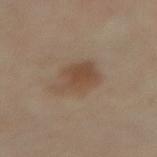No biopsy was performed on this lesion — it was imaged during a full skin examination and was not determined to be concerning. A 15 mm close-up tile from a total-body photography series done for melanoma screening. Located on the right thigh. A female subject aged around 50. Measured at roughly 5 mm in maximum diameter. The tile uses cross-polarized illumination.